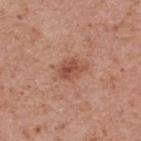• notes — imaged on a skin check; not biopsied